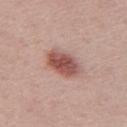This is a white-light tile.
The patient is a male about 55 years old.
A region of skin cropped from a whole-body photographic capture, roughly 15 mm wide.
About 4 mm across.
Located on the mid back.
Automated image analysis of the tile measured a footprint of about 10 mm², a shape eccentricity near 0.65, and a shape-asymmetry score of about 0.15 (0 = symmetric). It also reported an average lesion color of about L≈52 a*≈24 b*≈24 (CIELAB), about 14 CIELAB-L* units darker than the surrounding skin, and a lesion-to-skin contrast of about 9.5 (normalized; higher = more distinct). It also reported border irregularity of about 1.5 on a 0–10 scale and peripheral color asymmetry of about 1.